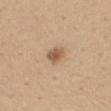Notes:
– workup — total-body-photography surveillance lesion; no biopsy
– patient — male, in their 40s
– automated metrics — an average lesion color of about L≈57 a*≈19 b*≈32 (CIELAB) and roughly 12 lightness units darker than nearby skin; border irregularity of about 2 on a 0–10 scale, a color-variation rating of about 3/10, and radial color variation of about 1; a classifier nevus-likeness of about 90/100 and lesion-presence confidence of about 100/100
– location — the mid back
– diameter — ~2.5 mm (longest diameter)
– imaging modality — ~15 mm tile from a whole-body skin photo
– tile lighting — white-light illumination